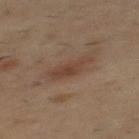biopsy_status: not biopsied; imaged during a skin examination
patient:
  sex: male
  age_approx: 55
lighting: cross-polarized
site: mid back
image:
  source: total-body photography crop
  field_of_view_mm: 15
lesion_size:
  long_diameter_mm_approx: 4.5